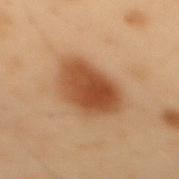Captured during whole-body skin photography for melanoma surveillance; the lesion was not biopsied. Approximately 6 mm at its widest. Located on the mid back. An algorithmic analysis of the crop reported a lesion–skin lightness drop of about 12. And it measured border irregularity of about 2 on a 0–10 scale, a within-lesion color-variation index near 4.5/10, and peripheral color asymmetry of about 1.5. A lesion tile, about 15 mm wide, cut from a 3D total-body photograph. A male subject, aged 53 to 57. Captured under cross-polarized illumination.